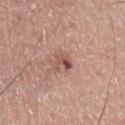| feature | finding |
|---|---|
| subject | male, aged 63–67 |
| tile lighting | white-light illumination |
| anatomic site | the left lower leg |
| diameter | ~2.5 mm (longest diameter) |
| image-analysis metrics | an area of roughly 4 mm², an eccentricity of roughly 0.65, and a shape-asymmetry score of about 0.3 (0 = symmetric); border irregularity of about 3.5 on a 0–10 scale, a within-lesion color-variation index near 8.5/10, and a peripheral color-asymmetry measure near 3 |
| acquisition | ~15 mm crop, total-body skin-cancer survey |